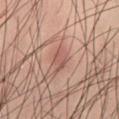Recorded during total-body skin imaging; not selected for excision or biopsy. A male subject, in their 40s. A 15 mm close-up extracted from a 3D total-body photography capture. The lesion is located on the lower back. An algorithmic analysis of the crop reported a lesion color around L≈53 a*≈22 b*≈25 in CIELAB, about 8 CIELAB-L* units darker than the surrounding skin, and a normalized lesion–skin contrast near 6. The analysis additionally found a within-lesion color-variation index near 1/10 and a peripheral color-asymmetry measure near 0. The recorded lesion diameter is about 2.5 mm.